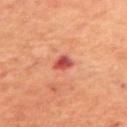Imaged during a routine full-body skin examination; the lesion was not biopsied and no histopathology is available. Captured under cross-polarized illumination. A 15 mm crop from a total-body photograph taken for skin-cancer surveillance. A male subject, about 70 years old. About 2.5 mm across. From the upper back.